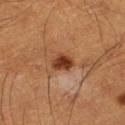<lesion>
<biopsy_status>not biopsied; imaged during a skin examination</biopsy_status>
<image>
  <source>total-body photography crop</source>
  <field_of_view_mm>15</field_of_view_mm>
</image>
<site>left thigh</site>
<patient>
  <sex>male</sex>
  <age_approx>60</age_approx>
</patient>
</lesion>